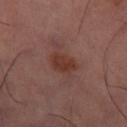Recorded during total-body skin imaging; not selected for excision or biopsy.
The recorded lesion diameter is about 3.5 mm.
A lesion tile, about 15 mm wide, cut from a 3D total-body photograph.
Located on the right thigh.
This is a cross-polarized tile.